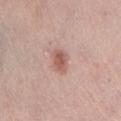Impression:
Imaged during a routine full-body skin examination; the lesion was not biopsied and no histopathology is available.
Clinical summary:
The lesion-visualizer software estimated an area of roughly 4.5 mm², a shape eccentricity near 0.7, and two-axis asymmetry of about 0.15. It also reported roughly 11 lightness units darker than nearby skin and a normalized border contrast of about 7.5. It also reported border irregularity of about 1.5 on a 0–10 scale and a within-lesion color-variation index near 3/10. The patient is a female roughly 65 years of age. Longest diameter approximately 2.5 mm. A lesion tile, about 15 mm wide, cut from a 3D total-body photograph. Located on the left lower leg.